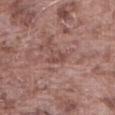{
  "biopsy_status": "not biopsied; imaged during a skin examination",
  "site": "abdomen",
  "automated_metrics": {
    "cielab_L": 48,
    "cielab_a": 21,
    "cielab_b": 23,
    "vs_skin_contrast_norm": 5.5
  },
  "lesion_size": {
    "long_diameter_mm_approx": 2.5
  },
  "image": {
    "source": "total-body photography crop",
    "field_of_view_mm": 15
  },
  "patient": {
    "sex": "male",
    "age_approx": 75
  },
  "lighting": "white-light"
}